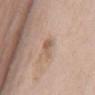Part of a total-body skin-imaging series; this lesion was reviewed on a skin check and was not flagged for biopsy. A 15 mm crop from a total-body photograph taken for skin-cancer surveillance. This is a white-light tile. On the mid back. A male patient in their mid- to late 60s. Longest diameter approximately 3.5 mm.